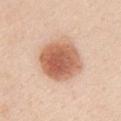  biopsy_status: not biopsied; imaged during a skin examination
  lesion_size:
    long_diameter_mm_approx: 5.5
  automated_metrics:
    area_mm2_approx: 24.0
    eccentricity: 0.4
    shape_asymmetry: 0.1
    cielab_L: 62
    cielab_a: 23
    cielab_b: 33
    vs_skin_darker_L: 16.0
    vs_skin_contrast_norm: 9.5
    border_irregularity_0_10: 1.0
    color_variation_0_10: 6.0
    nevus_likeness_0_100: 100
    lesion_detection_confidence_0_100: 100
  site: front of the torso
  patient:
    sex: female
    age_approx: 55
  lighting: white-light
  image:
    source: total-body photography crop
    field_of_view_mm: 15